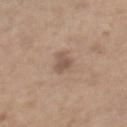  biopsy_status: not biopsied; imaged during a skin examination
  lighting: white-light
  lesion_size:
    long_diameter_mm_approx: 3.0
  site: chest
  image:
    source: total-body photography crop
    field_of_view_mm: 15
  patient:
    sex: female
    age_approx: 65